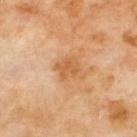A male subject roughly 70 years of age.
Located on the upper back.
A 15 mm close-up tile from a total-body photography series done for melanoma screening.
Imaged with cross-polarized lighting.